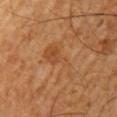| feature | finding |
|---|---|
| workup | imaged on a skin check; not biopsied |
| subject | male, in their 60s |
| location | the left upper arm |
| image source | 15 mm crop, total-body photography |